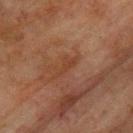Q: Was this lesion biopsied?
A: imaged on a skin check; not biopsied
Q: What are the patient's age and sex?
A: male, aged 73 to 77
Q: What lighting was used for the tile?
A: cross-polarized illumination
Q: How was this image acquired?
A: ~15 mm crop, total-body skin-cancer survey
Q: Where on the body is the lesion?
A: the upper back
Q: What did automated image analysis measure?
A: an area of roughly 3.5 mm² and two-axis asymmetry of about 0.6; about 4 CIELAB-L* units darker than the surrounding skin and a normalized lesion–skin contrast near 5; a border-irregularity rating of about 6/10 and radial color variation of about 0